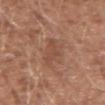Q: Was a biopsy performed?
A: total-body-photography surveillance lesion; no biopsy
Q: Where on the body is the lesion?
A: the left upper arm
Q: What is the imaging modality?
A: ~15 mm tile from a whole-body skin photo
Q: What are the patient's age and sex?
A: male, about 45 years old